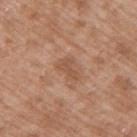No biopsy was performed on this lesion — it was imaged during a full skin examination and was not determined to be concerning.